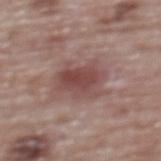Q: Was a biopsy performed?
A: total-body-photography surveillance lesion; no biopsy
Q: What is the lesion's diameter?
A: ≈5 mm
Q: What is the anatomic site?
A: the upper back
Q: Who is the patient?
A: female, aged 63–67
Q: What lighting was used for the tile?
A: white-light
Q: What kind of image is this?
A: 15 mm crop, total-body photography
Q: Automated lesion metrics?
A: two-axis asymmetry of about 0.25; a border-irregularity index near 3.5/10 and peripheral color asymmetry of about 1.5; a nevus-likeness score of about 20/100 and a detector confidence of about 100 out of 100 that the crop contains a lesion